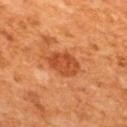biopsy status = no biopsy performed (imaged during a skin exam) | subject = male, in their mid- to late 60s | lesion size = about 4 mm | acquisition = ~15 mm tile from a whole-body skin photo | location = the upper back | illumination = cross-polarized illumination.